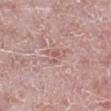Q: Is there a histopathology result?
A: catalogued during a skin exam; not biopsied
Q: Where on the body is the lesion?
A: the right lower leg
Q: Patient demographics?
A: male, in their 50s
Q: What kind of image is this?
A: ~15 mm crop, total-body skin-cancer survey